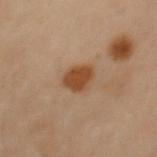Findings:
– biopsy status: imaged on a skin check; not biopsied
– automated lesion analysis: a nevus-likeness score of about 100/100
– image: ~15 mm crop, total-body skin-cancer survey
– patient: female, in their 60s
– tile lighting: cross-polarized
– body site: the right arm